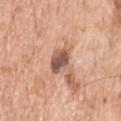follow-up=imaged on a skin check; not biopsied
acquisition=total-body-photography crop, ~15 mm field of view
location=the head or neck
subject=male, aged 68–72
diameter=≈4 mm
illumination=white-light